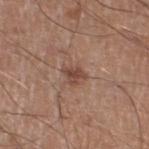Impression: The lesion was photographed on a routine skin check and not biopsied; there is no pathology result. Background: Automated image analysis of the tile measured an eccentricity of roughly 0.6 and two-axis asymmetry of about 0.45. It also reported a lesion color around L≈45 a*≈20 b*≈26 in CIELAB, a lesion–skin lightness drop of about 10, and a lesion-to-skin contrast of about 7.5 (normalized; higher = more distinct). The software also gave a classifier nevus-likeness of about 20/100 and lesion-presence confidence of about 100/100. The lesion is on the left lower leg. The tile uses white-light illumination. A region of skin cropped from a whole-body photographic capture, roughly 15 mm wide. Longest diameter approximately 2.5 mm. A male subject, about 20 years old.